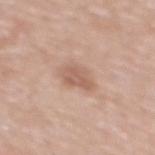Captured during whole-body skin photography for melanoma surveillance; the lesion was not biopsied.